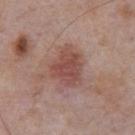Captured during whole-body skin photography for melanoma surveillance; the lesion was not biopsied.